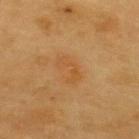Clinical impression:
The lesion was tiled from a total-body skin photograph and was not biopsied.
Acquisition and patient details:
Automated tile analysis of the lesion measured a border-irregularity rating of about 4.5/10, internal color variation of about 4 on a 0–10 scale, and peripheral color asymmetry of about 1.5. The software also gave a nevus-likeness score of about 5/100 and a lesion-detection confidence of about 100/100. The lesion's longest dimension is about 3.5 mm. The subject is a male aged approximately 60. Located on the back. The tile uses cross-polarized illumination. Cropped from a whole-body photographic skin survey; the tile spans about 15 mm.